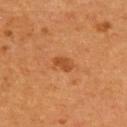No biopsy was performed on this lesion — it was imaged during a full skin examination and was not determined to be concerning. Captured under cross-polarized illumination. A male subject roughly 55 years of age. Measured at roughly 2.5 mm in maximum diameter. The lesion is on the upper back. Cropped from a total-body skin-imaging series; the visible field is about 15 mm.